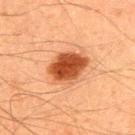workup: total-body-photography surveillance lesion; no biopsy | anatomic site: the upper back | tile lighting: cross-polarized | patient: male, aged 43–47 | lesion size: about 4.5 mm | imaging modality: ~15 mm crop, total-body skin-cancer survey.